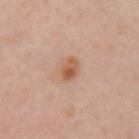follow-up = catalogued during a skin exam; not biopsied | patient = female, aged 38 to 42 | illumination = cross-polarized | automated metrics = a shape eccentricity near 0.75 and two-axis asymmetry of about 0.15; a mean CIELAB color near L≈56 a*≈22 b*≈33, roughly 10 lightness units darker than nearby skin, and a normalized border contrast of about 8.5 | diameter = about 3 mm | site = the left upper arm | acquisition = ~15 mm tile from a whole-body skin photo.